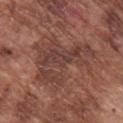Impression: The lesion was photographed on a routine skin check and not biopsied; there is no pathology result. Background: The lesion's longest dimension is about 7.5 mm. A male patient aged around 75. A roughly 15 mm field-of-view crop from a total-body skin photograph. The lesion is located on the right upper arm. An algorithmic analysis of the crop reported an average lesion color of about L≈41 a*≈21 b*≈24 (CIELAB), roughly 7 lightness units darker than nearby skin, and a normalized lesion–skin contrast near 6.